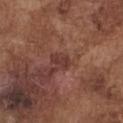biopsy status: imaged on a skin check; not biopsied | lighting: white-light illumination | imaging modality: ~15 mm tile from a whole-body skin photo | size: ≈2.5 mm | site: the chest | subject: male, approximately 75 years of age | TBP lesion metrics: a shape eccentricity near 0.9 and a symmetry-axis asymmetry near 0.3; a lesion–skin lightness drop of about 8 and a normalized border contrast of about 7; a nevus-likeness score of about 0/100 and a lesion-detection confidence of about 100/100.